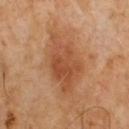Clinical impression:
Captured during whole-body skin photography for melanoma surveillance; the lesion was not biopsied.
Clinical summary:
A roughly 15 mm field-of-view crop from a total-body skin photograph. The subject is a male roughly 65 years of age. Captured under cross-polarized illumination.